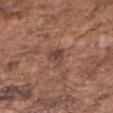Assessment:
The lesion was tiled from a total-body skin photograph and was not biopsied.
Image and clinical context:
The patient is a male aged approximately 75. Longest diameter approximately 3 mm. On the right upper arm. Cropped from a whole-body photographic skin survey; the tile spans about 15 mm. An algorithmic analysis of the crop reported a footprint of about 4 mm², an outline eccentricity of about 0.8 (0 = round, 1 = elongated), and a symmetry-axis asymmetry near 0.25. And it measured a mean CIELAB color near L≈43 a*≈21 b*≈25, a lesion–skin lightness drop of about 9, and a lesion-to-skin contrast of about 7.5 (normalized; higher = more distinct). It also reported a within-lesion color-variation index near 3/10 and peripheral color asymmetry of about 1. It also reported an automated nevus-likeness rating near 0 out of 100.